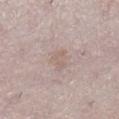Case summary:
– workup: total-body-photography surveillance lesion; no biopsy
– illumination: white-light
– TBP lesion metrics: an area of roughly 3.5 mm² and an outline eccentricity of about 0.8 (0 = round, 1 = elongated); border irregularity of about 5 on a 0–10 scale; a nevus-likeness score of about 0/100 and lesion-presence confidence of about 75/100
– acquisition: ~15 mm crop, total-body skin-cancer survey
– anatomic site: the right lower leg
– subject: female, aged approximately 50
– lesion size: ~3 mm (longest diameter)